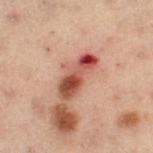The total-body-photography lesion software estimated a lesion area of about 8.5 mm², an eccentricity of roughly 0.95, and a symmetry-axis asymmetry near 0.25. The analysis additionally found radial color variation of about 3.
Approximately 5.5 mm at its widest.
A 15 mm crop from a total-body photograph taken for skin-cancer surveillance.
Captured under cross-polarized illumination.
On the left thigh.
A female subject, aged around 55.